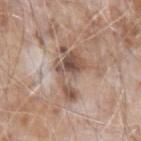Q: Was this lesion biopsied?
A: total-body-photography surveillance lesion; no biopsy
Q: What are the patient's age and sex?
A: male, in their mid-70s
Q: Where on the body is the lesion?
A: the left forearm
Q: How large is the lesion?
A: about 6 mm
Q: Automated lesion metrics?
A: a lesion area of about 12 mm², an outline eccentricity of about 0.85 (0 = round, 1 = elongated), and a shape-asymmetry score of about 0.55 (0 = symmetric); a border-irregularity index near 7.5/10, internal color variation of about 7 on a 0–10 scale, and radial color variation of about 2.5; lesion-presence confidence of about 55/100
Q: How was this image acquired?
A: ~15 mm tile from a whole-body skin photo